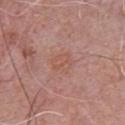Impression:
The lesion was tiled from a total-body skin photograph and was not biopsied.
Background:
A roughly 15 mm field-of-view crop from a total-body skin photograph. The lesion is located on the front of the torso. A male patient roughly 70 years of age.